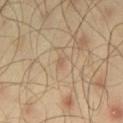Clinical impression: The lesion was tiled from a total-body skin photograph and was not biopsied. Acquisition and patient details: Longest diameter approximately 1 mm. Automated tile analysis of the lesion measured a lesion area of about 1 mm² and a shape eccentricity near 0.75. And it measured a border-irregularity index near 4/10, a color-variation rating of about 0/10, and peripheral color asymmetry of about 0. The lesion is on the left thigh. Imaged with cross-polarized lighting. Cropped from a total-body skin-imaging series; the visible field is about 15 mm. A male subject roughly 45 years of age.